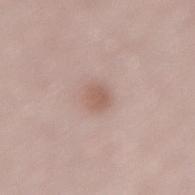Case summary:
• biopsy status: imaged on a skin check; not biopsied
• anatomic site: the lower back
• patient: male, aged 68 to 72
• illumination: white-light illumination
• automated lesion analysis: a border-irregularity rating of about 1.5/10, a within-lesion color-variation index near 1.5/10, and peripheral color asymmetry of about 0.5; a detector confidence of about 100 out of 100 that the crop contains a lesion
• lesion size: ≈2.5 mm
• image: 15 mm crop, total-body photography